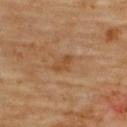Clinical impression: Recorded during total-body skin imaging; not selected for excision or biopsy. Background: Automated image analysis of the tile measured a footprint of about 3 mm², an outline eccentricity of about 0.85 (0 = round, 1 = elongated), and two-axis asymmetry of about 0.3. It also reported border irregularity of about 3 on a 0–10 scale and radial color variation of about 0.5. The analysis additionally found a classifier nevus-likeness of about 0/100 and a detector confidence of about 100 out of 100 that the crop contains a lesion. Approximately 3 mm at its widest. Captured under cross-polarized illumination. The lesion is located on the upper back. A 15 mm crop from a total-body photograph taken for skin-cancer surveillance. A female subject, approximately 60 years of age.